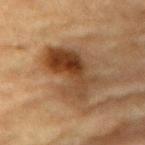| feature | finding |
|---|---|
| workup | imaged on a skin check; not biopsied |
| image | total-body-photography crop, ~15 mm field of view |
| site | the back |
| tile lighting | cross-polarized |
| TBP lesion metrics | a lesion area of about 20 mm², a shape eccentricity near 0.85, and a shape-asymmetry score of about 0.35 (0 = symmetric) |
| subject | male, in their mid-80s or older |
| lesion diameter | about 7 mm |